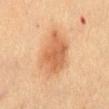  biopsy_status: not biopsied; imaged during a skin examination
  lesion_size:
    long_diameter_mm_approx: 6.0
  image:
    source: total-body photography crop
    field_of_view_mm: 15
  automated_metrics:
    eccentricity: 0.8
    shape_asymmetry: 0.2
    nevus_likeness_0_100: 95
    lesion_detection_confidence_0_100: 100
  patient:
    sex: female
    age_approx: 70
  site: lower back
  lighting: cross-polarized